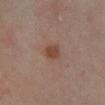{
  "biopsy_status": "not biopsied; imaged during a skin examination",
  "lighting": "cross-polarized",
  "image": {
    "source": "total-body photography crop",
    "field_of_view_mm": 15
  },
  "automated_metrics": {
    "area_mm2_approx": 4.5,
    "shape_asymmetry": 0.15,
    "cielab_L": 42,
    "cielab_a": 19,
    "cielab_b": 26,
    "lesion_detection_confidence_0_100": 100
  },
  "lesion_size": {
    "long_diameter_mm_approx": 2.5
  },
  "patient": {
    "sex": "female",
    "age_approx": 35
  },
  "site": "right lower leg"
}